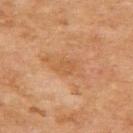<record>
  <biopsy_status>not biopsied; imaged during a skin examination</biopsy_status>
  <site>back</site>
  <patient>
    <sex>female</sex>
    <age_approx>60</age_approx>
  </patient>
  <lighting>cross-polarized</lighting>
  <image>
    <source>total-body photography crop</source>
    <field_of_view_mm>15</field_of_view_mm>
  </image>
  <lesion_size>
    <long_diameter_mm_approx>2.5</long_diameter_mm_approx>
  </lesion_size>
</record>